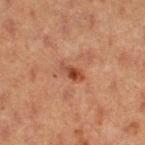The lesion was tiled from a total-body skin photograph and was not biopsied. Approximately 3 mm at its widest. A female patient roughly 40 years of age. A region of skin cropped from a whole-body photographic capture, roughly 15 mm wide. Automated tile analysis of the lesion measured an average lesion color of about L≈39 a*≈24 b*≈29 (CIELAB) and roughly 9 lightness units darker than nearby skin. And it measured a border-irregularity index near 3/10 and radial color variation of about 1. The analysis additionally found a classifier nevus-likeness of about 45/100 and a detector confidence of about 100 out of 100 that the crop contains a lesion. Located on the right thigh.